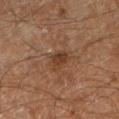Impression:
Recorded during total-body skin imaging; not selected for excision or biopsy.
Context:
The tile uses cross-polarized illumination. A close-up tile cropped from a whole-body skin photograph, about 15 mm across. The lesion is located on the left lower leg. A male subject approximately 60 years of age. The recorded lesion diameter is about 2.5 mm.